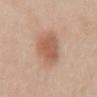biopsy_status: not biopsied; imaged during a skin examination
lighting: white-light
image:
  source: total-body photography crop
  field_of_view_mm: 15
patient:
  sex: male
  age_approx: 60
site: back
lesion_size:
  long_diameter_mm_approx: 4.5